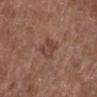Notes:
• workup · total-body-photography surveillance lesion; no biopsy
• tile lighting · white-light
• lesion diameter · ~3 mm (longest diameter)
• anatomic site · the left lower leg
• image source · ~15 mm crop, total-body skin-cancer survey
• subject · female, aged 78 to 82